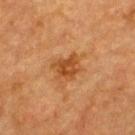This lesion was catalogued during total-body skin photography and was not selected for biopsy. The lesion is on the back. A female patient, roughly 55 years of age. Approximately 3 mm at its widest. Automated image analysis of the tile measured a lesion area of about 6 mm², an eccentricity of roughly 0.55, and a shape-asymmetry score of about 0.3 (0 = symmetric). This is a cross-polarized tile. Cropped from a total-body skin-imaging series; the visible field is about 15 mm.